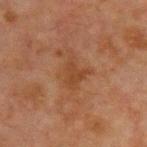follow-up: imaged on a skin check; not biopsied | anatomic site: the chest | illumination: cross-polarized | automated lesion analysis: an area of roughly 5.5 mm², an eccentricity of roughly 0.65, and two-axis asymmetry of about 0.4; a lesion color around L≈34 a*≈19 b*≈29 in CIELAB and a lesion-to-skin contrast of about 6 (normalized; higher = more distinct); an automated nevus-likeness rating near 0 out of 100 and lesion-presence confidence of about 100/100 | subject: male, approximately 65 years of age | image: ~15 mm crop, total-body skin-cancer survey.